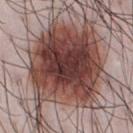The lesion was photographed on a routine skin check and not biopsied; there is no pathology result.
This image is a 15 mm lesion crop taken from a total-body photograph.
Longest diameter approximately 12 mm.
Located on the chest.
A male patient, about 35 years old.